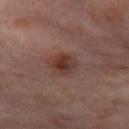notes = imaged on a skin check; not biopsied
body site = the right thigh
patient = female, aged 53 to 57
imaging modality = ~15 mm tile from a whole-body skin photo
tile lighting = cross-polarized illumination
lesion diameter = ~3 mm (longest diameter)